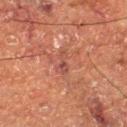diameter: ~3.5 mm (longest diameter) | site: the right thigh | subject: male, aged around 75 | image-analysis metrics: an outline eccentricity of about 0.95 (0 = round, 1 = elongated) and a shape-asymmetry score of about 0.5 (0 = symmetric); a lesion-to-skin contrast of about 6 (normalized; higher = more distinct); a border-irregularity rating of about 6/10, internal color variation of about 1 on a 0–10 scale, and a peripheral color-asymmetry measure near 0; a nevus-likeness score of about 0/100 and a lesion-detection confidence of about 85/100 | illumination: cross-polarized | image source: ~15 mm crop, total-body skin-cancer survey.